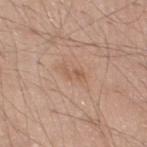This lesion was catalogued during total-body skin photography and was not selected for biopsy. The tile uses white-light illumination. Longest diameter approximately 2.5 mm. A 15 mm crop from a total-body photograph taken for skin-cancer surveillance. The subject is a male aged approximately 60. On the leg. The total-body-photography lesion software estimated a border-irregularity index near 7/10 and a within-lesion color-variation index near 0/10. And it measured a nevus-likeness score of about 0/100 and a detector confidence of about 100 out of 100 that the crop contains a lesion.